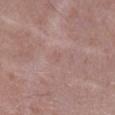Impression: Imaged during a routine full-body skin examination; the lesion was not biopsied and no histopathology is available. Background: A male patient approximately 70 years of age. The lesion is located on the right lower leg. A close-up tile cropped from a whole-body skin photograph, about 15 mm across.